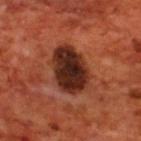| feature | finding |
|---|---|
| workup | catalogued during a skin exam; not biopsied |
| patient | male, aged approximately 70 |
| location | the back |
| TBP lesion metrics | an average lesion color of about L≈27 a*≈24 b*≈27 (CIELAB), roughly 16 lightness units darker than nearby skin, and a lesion-to-skin contrast of about 15 (normalized; higher = more distinct); a nevus-likeness score of about 10/100 and a lesion-detection confidence of about 100/100 |
| image source | ~15 mm crop, total-body skin-cancer survey |
| tile lighting | cross-polarized |